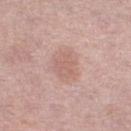Assessment: Imaged during a routine full-body skin examination; the lesion was not biopsied and no histopathology is available. Context: A close-up tile cropped from a whole-body skin photograph, about 15 mm across. The subject is a female aged 58 to 62. Located on the right lower leg. The tile uses white-light illumination. The total-body-photography lesion software estimated an area of roughly 11 mm² and a symmetry-axis asymmetry near 0.25. It also reported an average lesion color of about L≈62 a*≈20 b*≈24 (CIELAB) and roughly 7 lightness units darker than nearby skin. The analysis additionally found border irregularity of about 2.5 on a 0–10 scale. The analysis additionally found a detector confidence of about 100 out of 100 that the crop contains a lesion.